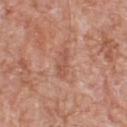acquisition — total-body-photography crop, ~15 mm field of view; anatomic site — the back; illumination — white-light; patient — male, aged approximately 80; lesion size — ~3.5 mm (longest diameter).